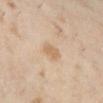workup = total-body-photography surveillance lesion; no biopsy | location = the left lower leg | subject = female, roughly 45 years of age | illumination = cross-polarized | TBP lesion metrics = an average lesion color of about L≈65 a*≈16 b*≈34 (CIELAB), roughly 8 lightness units darker than nearby skin, and a normalized border contrast of about 6; a classifier nevus-likeness of about 35/100 and lesion-presence confidence of about 100/100 | acquisition = ~15 mm tile from a whole-body skin photo.